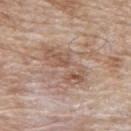This lesion was catalogued during total-body skin photography and was not selected for biopsy.
A region of skin cropped from a whole-body photographic capture, roughly 15 mm wide.
On the back.
A male subject in their 80s.